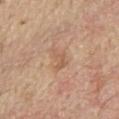| feature | finding |
|---|---|
| workup | imaged on a skin check; not biopsied |
| tile lighting | white-light |
| body site | the abdomen |
| image-analysis metrics | a mean CIELAB color near L≈58 a*≈20 b*≈32 and about 7 CIELAB-L* units darker than the surrounding skin; a border-irregularity index near 4.5/10, a within-lesion color-variation index near 1/10, and radial color variation of about 0.5; a lesion-detection confidence of about 100/100 |
| subject | male, aged approximately 70 |
| acquisition | ~15 mm tile from a whole-body skin photo |